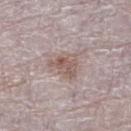Captured during whole-body skin photography for melanoma surveillance; the lesion was not biopsied. The lesion-visualizer software estimated a lesion area of about 6.5 mm². And it measured an average lesion color of about L≈55 a*≈16 b*≈21 (CIELAB) and a lesion-to-skin contrast of about 7 (normalized; higher = more distinct). The analysis additionally found a border-irregularity rating of about 4.5/10, a color-variation rating of about 4/10, and a peripheral color-asymmetry measure near 1.5. The tile uses white-light illumination. A female subject aged around 65. About 3.5 mm across. Cropped from a total-body skin-imaging series; the visible field is about 15 mm. Located on the right lower leg.